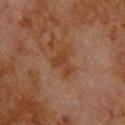<lesion>
  <biopsy_status>not biopsied; imaged during a skin examination</biopsy_status>
  <patient>
    <sex>male</sex>
    <age_approx>80</age_approx>
  </patient>
  <automated_metrics>
    <area_mm2_approx>5.0</area_mm2_approx>
    <eccentricity>0.7</eccentricity>
    <shape_asymmetry>0.65</shape_asymmetry>
    <cielab_L>31</cielab_L>
    <cielab_a>19</cielab_a>
    <cielab_b>27</cielab_b>
    <vs_skin_darker_L>5.0</vs_skin_darker_L>
    <nevus_likeness_0_100>0</nevus_likeness_0_100>
    <lesion_detection_confidence_0_100>100</lesion_detection_confidence_0_100>
  </automated_metrics>
  <image>
    <source>total-body photography crop</source>
    <field_of_view_mm>15</field_of_view_mm>
  </image>
  <lesion_size>
    <long_diameter_mm_approx>3.5</long_diameter_mm_approx>
  </lesion_size>
  <site>upper back</site>
</lesion>